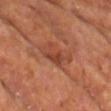Notes:
* biopsy status: catalogued during a skin exam; not biopsied
* automated lesion analysis: an area of roughly 7 mm², a shape eccentricity near 0.75, and two-axis asymmetry of about 0.25; a border-irregularity index near 4/10, a color-variation rating of about 4/10, and peripheral color asymmetry of about 1.5; an automated nevus-likeness rating near 55 out of 100
* image: 15 mm crop, total-body photography
* tile lighting: cross-polarized illumination
* subject: male, aged approximately 60
* site: the upper back
* lesion diameter: ~4 mm (longest diameter)